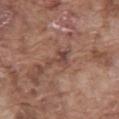biopsy status: total-body-photography surveillance lesion; no biopsy | lighting: white-light | size: ~3 mm (longest diameter) | image-analysis metrics: a footprint of about 4 mm², a shape eccentricity near 0.85, and a symmetry-axis asymmetry near 0.6; an average lesion color of about L≈44 a*≈19 b*≈24 (CIELAB) and a normalized lesion–skin contrast near 6.5; border irregularity of about 7 on a 0–10 scale and internal color variation of about 3.5 on a 0–10 scale | subject: male, aged 73 to 77 | anatomic site: the abdomen | acquisition: ~15 mm tile from a whole-body skin photo.